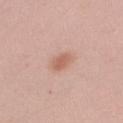This lesion was catalogued during total-body skin photography and was not selected for biopsy. A 15 mm close-up extracted from a 3D total-body photography capture. A female patient, aged 28–32. The lesion is located on the right upper arm.